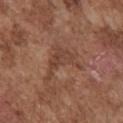follow-up: imaged on a skin check; not biopsied
location: the front of the torso
image-analysis metrics: an area of roughly 7.5 mm², a shape eccentricity near 0.75, and a shape-asymmetry score of about 0.6 (0 = symmetric); a border-irregularity rating of about 8.5/10 and a peripheral color-asymmetry measure near 1; a classifier nevus-likeness of about 0/100 and a lesion-detection confidence of about 95/100
subject: male, aged approximately 75
lighting: white-light illumination
image: ~15 mm crop, total-body skin-cancer survey